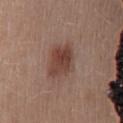Clinical impression: Part of a total-body skin-imaging series; this lesion was reviewed on a skin check and was not flagged for biopsy.